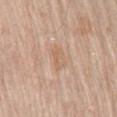| field | value |
|---|---|
| workup | imaged on a skin check; not biopsied |
| lesion size | about 3 mm |
| site | the mid back |
| lighting | white-light illumination |
| imaging modality | 15 mm crop, total-body photography |
| patient | male, aged 68–72 |